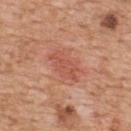{"biopsy_status": "not biopsied; imaged during a skin examination", "lesion_size": {"long_diameter_mm_approx": 4.5}, "site": "back", "automated_metrics": {"cielab_L": 55, "cielab_a": 28, "cielab_b": 31, "vs_skin_contrast_norm": 5.0, "nevus_likeness_0_100": 5, "lesion_detection_confidence_0_100": 100}, "lighting": "white-light", "patient": {"sex": "male", "age_approx": 60}, "image": {"source": "total-body photography crop", "field_of_view_mm": 15}}